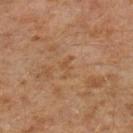Findings:
* lesion diameter: ~2.5 mm (longest diameter)
* subject: male, approximately 30 years of age
* image-analysis metrics: a mean CIELAB color near L≈49 a*≈19 b*≈34, roughly 5 lightness units darker than nearby skin, and a lesion-to-skin contrast of about 5 (normalized; higher = more distinct); a nevus-likeness score of about 0/100 and a lesion-detection confidence of about 100/100
* illumination: cross-polarized illumination
* acquisition: ~15 mm crop, total-body skin-cancer survey
* body site: the left lower leg